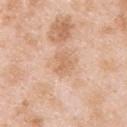notes — imaged on a skin check; not biopsied | tile lighting — white-light illumination | acquisition — ~15 mm crop, total-body skin-cancer survey | patient — male, in their mid-20s | location — the back | lesion diameter — about 3 mm | automated metrics — a footprint of about 5 mm², an eccentricity of roughly 0.7, and a symmetry-axis asymmetry near 0.35; a peripheral color-asymmetry measure near 0.5; an automated nevus-likeness rating near 0 out of 100 and a lesion-detection confidence of about 100/100.